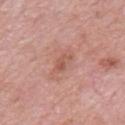Impression:
The lesion was photographed on a routine skin check and not biopsied; there is no pathology result.
Context:
The lesion is on the chest. The subject is a male in their mid-50s. A close-up tile cropped from a whole-body skin photograph, about 15 mm across. The recorded lesion diameter is about 2.5 mm. The total-body-photography lesion software estimated a footprint of about 3 mm², an outline eccentricity of about 0.85 (0 = round, 1 = elongated), and two-axis asymmetry of about 0.4. And it measured an average lesion color of about L≈55 a*≈24 b*≈29 (CIELAB), roughly 8 lightness units darker than nearby skin, and a lesion-to-skin contrast of about 6 (normalized; higher = more distinct). It also reported a border-irregularity rating of about 4/10, a color-variation rating of about 1/10, and peripheral color asymmetry of about 0. It also reported an automated nevus-likeness rating near 0 out of 100 and a lesion-detection confidence of about 100/100. This is a white-light tile.